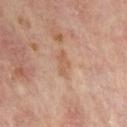– notes: total-body-photography surveillance lesion; no biopsy
– illumination: cross-polarized
– subject: in their mid-50s
– image: ~15 mm crop, total-body skin-cancer survey
– site: the right upper arm